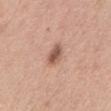  biopsy_status: not biopsied; imaged during a skin examination
  site: head or neck
  automated_metrics:
    area_mm2_approx: 4.0
    eccentricity: 0.8
    shape_asymmetry: 0.25
    color_variation_0_10: 2.5
    peripheral_color_asymmetry: 1.0
    nevus_likeness_0_100: 85
    lesion_detection_confidence_0_100: 100
  patient:
    sex: female
    age_approx: 40
  image:
    source: total-body photography crop
    field_of_view_mm: 15
  lighting: white-light
  lesion_size:
    long_diameter_mm_approx: 3.0